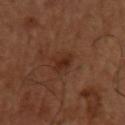Captured during whole-body skin photography for melanoma surveillance; the lesion was not biopsied. Captured under cross-polarized illumination. The lesion is on the arm. The total-body-photography lesion software estimated a footprint of about 5 mm² and two-axis asymmetry of about 0.25. The software also gave roughly 7 lightness units darker than nearby skin and a normalized border contrast of about 7. A male patient approximately 50 years of age. A 15 mm close-up extracted from a 3D total-body photography capture. About 3.5 mm across.